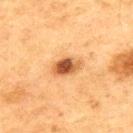workup — no biopsy performed (imaged during a skin exam); size — about 3.5 mm; image source — 15 mm crop, total-body photography; patient — male, aged approximately 75; body site — the upper back; tile lighting — cross-polarized illumination.